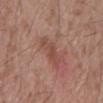Acquisition and patient details: A roughly 15 mm field-of-view crop from a total-body skin photograph. The lesion is on the mid back. A male patient, in their 60s. The lesion's longest dimension is about 4 mm.